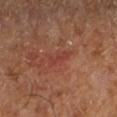Q: Is there a histopathology result?
A: no biopsy performed (imaged during a skin exam)
Q: What is the lesion's diameter?
A: about 3 mm
Q: What is the imaging modality?
A: ~15 mm tile from a whole-body skin photo
Q: Automated lesion metrics?
A: a footprint of about 3.5 mm², an outline eccentricity of about 0.9 (0 = round, 1 = elongated), and two-axis asymmetry of about 0.25; a border-irregularity rating of about 3.5/10, internal color variation of about 0 on a 0–10 scale, and a peripheral color-asymmetry measure near 0
Q: Patient demographics?
A: in their mid- to late 60s
Q: Lesion location?
A: the leg
Q: Illumination type?
A: cross-polarized